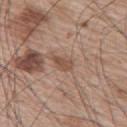  patient:
    sex: male
    age_approx: 65
  image:
    source: total-body photography crop
    field_of_view_mm: 15
  site: upper back